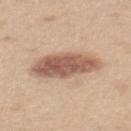follow-up: total-body-photography surveillance lesion; no biopsy
lighting: white-light illumination
TBP lesion metrics: a mean CIELAB color near L≈57 a*≈20 b*≈28, about 15 CIELAB-L* units darker than the surrounding skin, and a normalized lesion–skin contrast near 9.5
lesion diameter: ≈7 mm
acquisition: 15 mm crop, total-body photography
anatomic site: the back
subject: female, in their mid- to late 40s